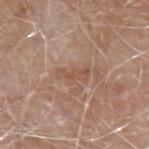Image and clinical context:
A roughly 15 mm field-of-view crop from a total-body skin photograph. The lesion is on the left forearm. Imaged with white-light lighting. The lesion-visualizer software estimated a lesion area of about 5 mm², an eccentricity of roughly 0.9, and two-axis asymmetry of about 0.5. It also reported a lesion–skin lightness drop of about 6 and a normalized border contrast of about 5. A male subject, aged 73–77. Longest diameter approximately 3.5 mm.